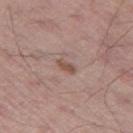Clinical impression:
This lesion was catalogued during total-body skin photography and was not selected for biopsy.
Context:
The lesion-visualizer software estimated a footprint of about 3 mm², an outline eccentricity of about 0.85 (0 = round, 1 = elongated), and a symmetry-axis asymmetry near 0.3. It also reported a border-irregularity rating of about 3/10 and a within-lesion color-variation index near 1/10. A male patient, aged approximately 55. The lesion's longest dimension is about 2.5 mm. The tile uses white-light illumination. This image is a 15 mm lesion crop taken from a total-body photograph. On the leg.